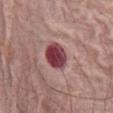  biopsy_status: not biopsied; imaged during a skin examination
  image:
    source: total-body photography crop
    field_of_view_mm: 15
  lesion_size:
    long_diameter_mm_approx: 3.5
  site: abdomen
  patient:
    sex: female
    age_approx: 65
  lighting: white-light